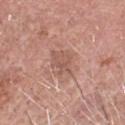Assessment: Imaged during a routine full-body skin examination; the lesion was not biopsied and no histopathology is available. Image and clinical context: A male patient in their 60s. The lesion is located on the head or neck. A 15 mm close-up tile from a total-body photography series done for melanoma screening.